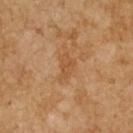{"biopsy_status": "not biopsied; imaged during a skin examination", "lighting": "cross-polarized", "patient": {"sex": "male", "age_approx": 65}, "lesion_size": {"long_diameter_mm_approx": 3.5}, "image": {"source": "total-body photography crop", "field_of_view_mm": 15}}